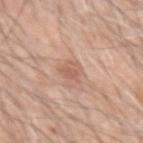No biopsy was performed on this lesion — it was imaged during a full skin examination and was not determined to be concerning. A 15 mm crop from a total-body photograph taken for skin-cancer surveillance. Automated image analysis of the tile measured an area of roughly 4.5 mm², a shape eccentricity near 0.5, and a shape-asymmetry score of about 0.35 (0 = symmetric). The analysis additionally found a lesion color around L≈60 a*≈20 b*≈29 in CIELAB, roughly 8 lightness units darker than nearby skin, and a normalized border contrast of about 5.5. The software also gave a border-irregularity index near 3.5/10, a within-lesion color-variation index near 2.5/10, and a peripheral color-asymmetry measure near 1. It also reported an automated nevus-likeness rating near 0 out of 100 and lesion-presence confidence of about 100/100. The lesion's longest dimension is about 2.5 mm. Imaged with white-light lighting. A male subject roughly 60 years of age. The lesion is located on the left upper arm.